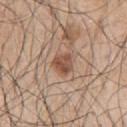Captured during whole-body skin photography for melanoma surveillance; the lesion was not biopsied. Automated tile analysis of the lesion measured a lesion area of about 5 mm² and a shape-asymmetry score of about 0.25 (0 = symmetric). The software also gave a border-irregularity rating of about 2/10, internal color variation of about 4 on a 0–10 scale, and a peripheral color-asymmetry measure near 1.5. The analysis additionally found a nevus-likeness score of about 90/100. Cropped from a total-body skin-imaging series; the visible field is about 15 mm. The lesion is on the left upper arm. Longest diameter approximately 2.5 mm. A male patient aged 68–72.